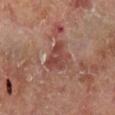{"biopsy_status": "not biopsied; imaged during a skin examination", "image": {"source": "total-body photography crop", "field_of_view_mm": 15}, "patient": {"sex": "male", "age_approx": 70}, "site": "leg", "lighting": "cross-polarized", "lesion_size": {"long_diameter_mm_approx": 4.0}}